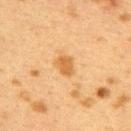<record>
<biopsy_status>not biopsied; imaged during a skin examination</biopsy_status>
<site>upper back</site>
<image>
  <source>total-body photography crop</source>
  <field_of_view_mm>15</field_of_view_mm>
</image>
<patient>
  <sex>female</sex>
  <age_approx>40</age_approx>
</patient>
<lighting>cross-polarized</lighting>
<lesion_size>
  <long_diameter_mm_approx>2.5</long_diameter_mm_approx>
</lesion_size>
</record>